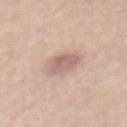Findings:
– workup · total-body-photography surveillance lesion; no biopsy
– size · ≈3.5 mm
– image · total-body-photography crop, ~15 mm field of view
– lighting · white-light
– site · the back
– TBP lesion metrics · border irregularity of about 1.5 on a 0–10 scale; an automated nevus-likeness rating near 10 out of 100 and lesion-presence confidence of about 100/100
– subject · male, aged 58–62